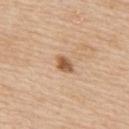{
  "image": {
    "source": "total-body photography crop",
    "field_of_view_mm": 15
  },
  "site": "upper back",
  "patient": {
    "sex": "male",
    "age_approx": 60
  }
}